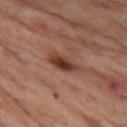| key | value |
|---|---|
| biopsy status | catalogued during a skin exam; not biopsied |
| location | the left thigh |
| imaging modality | 15 mm crop, total-body photography |
| lesion diameter | ≈4 mm |
| subject | female, in their mid- to late 50s |